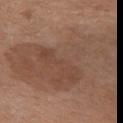Recorded during total-body skin imaging; not selected for excision or biopsy. Measured at roughly 14 mm in maximum diameter. A lesion tile, about 15 mm wide, cut from a 3D total-body photograph. Automated tile analysis of the lesion measured an eccentricity of roughly 0.9 and a symmetry-axis asymmetry near 0.4. And it measured a lesion color around L≈47 a*≈18 b*≈27 in CIELAB, a lesion–skin lightness drop of about 7, and a normalized border contrast of about 5.5. Captured under white-light illumination. The lesion is on the chest. A female patient, in their mid- to late 50s.